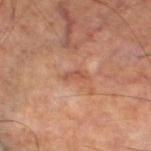Q: How was this image acquired?
A: total-body-photography crop, ~15 mm field of view
Q: What are the patient's age and sex?
A: male, in their mid- to late 50s
Q: Where on the body is the lesion?
A: the left lower leg